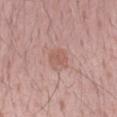Recorded during total-body skin imaging; not selected for excision or biopsy. The total-body-photography lesion software estimated a footprint of about 4 mm² and an eccentricity of roughly 0.7. The software also gave a mean CIELAB color near L≈57 a*≈23 b*≈25 and a lesion–skin lightness drop of about 7. And it measured a border-irregularity rating of about 2.5/10 and a color-variation rating of about 1/10. And it measured an automated nevus-likeness rating near 20 out of 100 and a lesion-detection confidence of about 100/100. A male subject approximately 25 years of age. This is a white-light tile. A 15 mm crop from a total-body photograph taken for skin-cancer surveillance. The lesion's longest dimension is about 2.5 mm. The lesion is located on the right forearm.